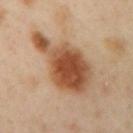Q: Was this lesion biopsied?
A: catalogued during a skin exam; not biopsied
Q: What is the imaging modality?
A: ~15 mm tile from a whole-body skin photo
Q: Who is the patient?
A: female, aged 38 to 42
Q: Illumination type?
A: cross-polarized illumination
Q: Automated lesion metrics?
A: an area of roughly 30 mm², an eccentricity of roughly 0.8, and a symmetry-axis asymmetry near 0.35; a border-irregularity index near 4/10 and radial color variation of about 2; a classifier nevus-likeness of about 100/100
Q: What is the anatomic site?
A: the left arm